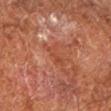| field | value |
|---|---|
| notes | imaged on a skin check; not biopsied |
| subject | male, about 65 years old |
| imaging modality | ~15 mm crop, total-body skin-cancer survey |
| body site | the left lower leg |
| size | ≈3.5 mm |
| lighting | cross-polarized illumination |